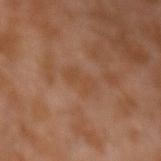Case summary:
– workup — catalogued during a skin exam; not biopsied
– image — ~15 mm crop, total-body skin-cancer survey
– automated metrics — an eccentricity of roughly 0.9 and a shape-asymmetry score of about 0.35 (0 = symmetric); a mean CIELAB color near L≈44 a*≈20 b*≈32, about 5 CIELAB-L* units darker than the surrounding skin, and a lesion-to-skin contrast of about 4.5 (normalized; higher = more distinct); border irregularity of about 4.5 on a 0–10 scale, a within-lesion color-variation index near 1/10, and a peripheral color-asymmetry measure near 0.5; a nevus-likeness score of about 0/100 and a detector confidence of about 100 out of 100 that the crop contains a lesion
– patient — male, about 30 years old
– size — ~3.5 mm (longest diameter)
– anatomic site — the left upper arm
– illumination — cross-polarized illumination